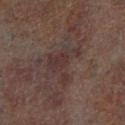Notes:
- biopsy status · imaged on a skin check; not biopsied
- image · total-body-photography crop, ~15 mm field of view
- subject · male, aged around 60
- lighting · cross-polarized
- lesion diameter · ~5 mm (longest diameter)
- anatomic site · the right lower leg